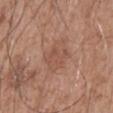Captured during whole-body skin photography for melanoma surveillance; the lesion was not biopsied.
About 4 mm across.
A 15 mm close-up tile from a total-body photography series done for melanoma screening.
On the mid back.
A male subject aged 53 to 57.
Imaged with white-light lighting.